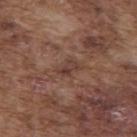patient — male, in their mid-70s | site — the upper back | tile lighting — white-light | image — ~15 mm tile from a whole-body skin photo | lesion size — ~2.5 mm (longest diameter).